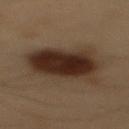notes = total-body-photography surveillance lesion; no biopsy | acquisition = total-body-photography crop, ~15 mm field of view | body site = the mid back | TBP lesion metrics = a footprint of about 29 mm², a shape eccentricity near 0.75, and two-axis asymmetry of about 0.2; a classifier nevus-likeness of about 95/100 | tile lighting = cross-polarized | subject = male, about 55 years old | size = about 7.5 mm.